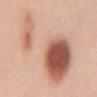follow-up — no biopsy performed (imaged during a skin exam) | subject — female, roughly 40 years of age | automated metrics — a footprint of about 70 mm², an eccentricity of roughly 0.85, and two-axis asymmetry of about 0.55 | lighting — white-light illumination | image — 15 mm crop, total-body photography | lesion size — ~14 mm (longest diameter) | location — the back.